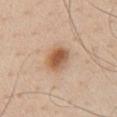Captured during whole-body skin photography for melanoma surveillance; the lesion was not biopsied. On the chest. The tile uses white-light illumination. A male subject aged around 60. A 15 mm close-up extracted from a 3D total-body photography capture. About 3.5 mm across.